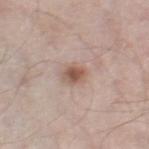body site = the right thigh; diameter = ≈3 mm; imaging modality = ~15 mm tile from a whole-body skin photo; TBP lesion metrics = lesion-presence confidence of about 100/100; patient = male, aged 63 to 67.